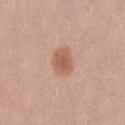workup: no biopsy performed (imaged during a skin exam)
body site: the right thigh
image-analysis metrics: a border-irregularity rating of about 2/10, internal color variation of about 2 on a 0–10 scale, and a peripheral color-asymmetry measure near 0.5; a nevus-likeness score of about 95/100 and a lesion-detection confidence of about 100/100
acquisition: 15 mm crop, total-body photography
lesion diameter: ≈3 mm
patient: female, roughly 20 years of age